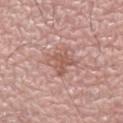Clinical impression: The lesion was photographed on a routine skin check and not biopsied; there is no pathology result. Clinical summary: A close-up tile cropped from a whole-body skin photograph, about 15 mm across. An algorithmic analysis of the crop reported a symmetry-axis asymmetry near 0.2. The software also gave a lesion color around L≈56 a*≈22 b*≈26 in CIELAB, roughly 8 lightness units darker than nearby skin, and a lesion-to-skin contrast of about 6 (normalized; higher = more distinct). And it measured a border-irregularity index near 3/10 and a within-lesion color-variation index near 3.5/10. Imaged with white-light lighting. From the left leg. A male patient, about 70 years old. Measured at roughly 3 mm in maximum diameter.